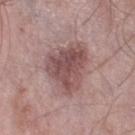The lesion was photographed on a routine skin check and not biopsied; there is no pathology result.